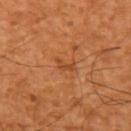lesion diameter: about 2.5 mm; body site: the left upper arm; acquisition: ~15 mm crop, total-body skin-cancer survey; tile lighting: cross-polarized; patient: aged around 65.